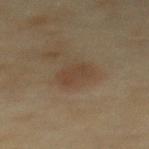biopsy status: imaged on a skin check; not biopsied
site: the upper back
image: 15 mm crop, total-body photography
tile lighting: cross-polarized
patient: female, approximately 60 years of age